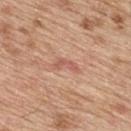Part of a total-body skin-imaging series; this lesion was reviewed on a skin check and was not flagged for biopsy.
The lesion is on the upper back.
The recorded lesion diameter is about 3 mm.
This is a white-light tile.
A male patient aged approximately 70.
A close-up tile cropped from a whole-body skin photograph, about 15 mm across.